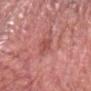Imaged during a routine full-body skin examination; the lesion was not biopsied and no histopathology is available.
A 15 mm crop from a total-body photograph taken for skin-cancer surveillance.
The lesion is located on the head or neck.
The patient is a male roughly 60 years of age.
Captured under white-light illumination.